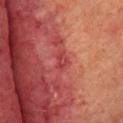Clinical impression: The lesion was tiled from a total-body skin photograph and was not biopsied. Image and clinical context: A 15 mm crop from a total-body photograph taken for skin-cancer surveillance. A female patient, in their mid-50s. The lesion's longest dimension is about 2.5 mm. Located on the front of the torso. Automated tile analysis of the lesion measured an outline eccentricity of about 0.8 (0 = round, 1 = elongated). And it measured a classifier nevus-likeness of about 0/100 and a detector confidence of about 75 out of 100 that the crop contains a lesion.